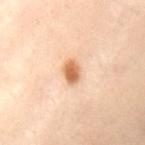Case summary:
• subject — female, aged approximately 60
• diameter — about 3 mm
• image — 15 mm crop, total-body photography
• lighting — cross-polarized
• anatomic site — the abdomen